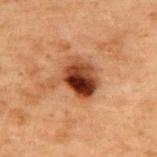Q: Was a biopsy performed?
A: no biopsy performed (imaged during a skin exam)
Q: What are the patient's age and sex?
A: male, aged 68 to 72
Q: Where on the body is the lesion?
A: the upper back
Q: How was the tile lit?
A: cross-polarized
Q: What did automated image analysis measure?
A: an area of roughly 14 mm² and a symmetry-axis asymmetry near 0.4; about 15 CIELAB-L* units darker than the surrounding skin and a normalized lesion–skin contrast near 12.5; an automated nevus-likeness rating near 100 out of 100
Q: What is the imaging modality?
A: 15 mm crop, total-body photography